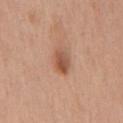| field | value |
|---|---|
| notes | total-body-photography surveillance lesion; no biopsy |
| patient | male, roughly 60 years of age |
| automated metrics | a lesion area of about 5.5 mm², a shape eccentricity near 0.75, and a shape-asymmetry score of about 0.15 (0 = symmetric); an automated nevus-likeness rating near 85 out of 100 and a lesion-detection confidence of about 100/100 |
| lighting | white-light illumination |
| lesion size | ≈3.5 mm |
| image | ~15 mm crop, total-body skin-cancer survey |
| anatomic site | the chest |